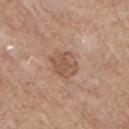Q: Is there a histopathology result?
A: imaged on a skin check; not biopsied
Q: How large is the lesion?
A: ≈3 mm
Q: What lighting was used for the tile?
A: white-light
Q: What kind of image is this?
A: ~15 mm tile from a whole-body skin photo
Q: What did automated image analysis measure?
A: an area of roughly 8.5 mm², an outline eccentricity of about 0.15 (0 = round, 1 = elongated), and two-axis asymmetry of about 0.2; a mean CIELAB color near L≈54 a*≈19 b*≈29, about 9 CIELAB-L* units darker than the surrounding skin, and a normalized border contrast of about 6
Q: Where on the body is the lesion?
A: the right upper arm
Q: Who is the patient?
A: male, roughly 70 years of age